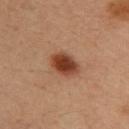Imaged during a routine full-body skin examination; the lesion was not biopsied and no histopathology is available. From the back. A female subject, in their mid- to late 30s. Captured under cross-polarized illumination. Longest diameter approximately 3.5 mm. A 15 mm crop from a total-body photograph taken for skin-cancer surveillance.